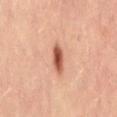Imaged with cross-polarized lighting.
On the lower back.
Approximately 3.5 mm at its widest.
This image is a 15 mm lesion crop taken from a total-body photograph.
An algorithmic analysis of the crop reported internal color variation of about 4.5 on a 0–10 scale and a peripheral color-asymmetry measure near 1.
A female patient aged around 35.